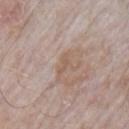The lesion was photographed on a routine skin check and not biopsied; there is no pathology result.
Captured under white-light illumination.
Cropped from a whole-body photographic skin survey; the tile spans about 15 mm.
The subject is a male aged 63–67.
Approximately 3 mm at its widest.
The lesion is located on the left upper arm.
The lesion-visualizer software estimated border irregularity of about 4 on a 0–10 scale, a color-variation rating of about 0/10, and radial color variation of about 0.